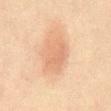The lesion was tiled from a total-body skin photograph and was not biopsied. From the abdomen. The tile uses cross-polarized illumination. An algorithmic analysis of the crop reported an eccentricity of roughly 0.75 and a shape-asymmetry score of about 0.2 (0 = symmetric). It also reported an automated nevus-likeness rating near 65 out of 100. A 15 mm crop from a total-body photograph taken for skin-cancer surveillance. The subject is a male aged 58 to 62.